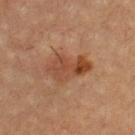The lesion was photographed on a routine skin check and not biopsied; there is no pathology result. The lesion-visualizer software estimated an automated nevus-likeness rating near 50 out of 100 and a lesion-detection confidence of about 100/100. A 15 mm close-up extracted from a 3D total-body photography capture. Located on the back. The subject is a male about 65 years old. Imaged with cross-polarized lighting.